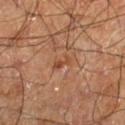The lesion was photographed on a routine skin check and not biopsied; there is no pathology result.
A male patient, aged around 60.
On the right lower leg.
Cropped from a whole-body photographic skin survey; the tile spans about 15 mm.
Longest diameter approximately 2.5 mm.